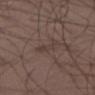The lesion was tiled from a total-body skin photograph and was not biopsied.
The patient is a male aged approximately 40.
A roughly 15 mm field-of-view crop from a total-body skin photograph.
Longest diameter approximately 3 mm.
The lesion is located on the right lower leg.